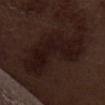<lesion>
  <biopsy_status>not biopsied; imaged during a skin examination</biopsy_status>
  <image>
    <source>total-body photography crop</source>
    <field_of_view_mm>15</field_of_view_mm>
  </image>
  <patient>
    <sex>male</sex>
    <age_approx>70</age_approx>
  </patient>
  <site>abdomen</site>
  <lesion_size>
    <long_diameter_mm_approx>8.5</long_diameter_mm_approx>
  </lesion_size>
</lesion>